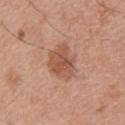Findings:
– notes — catalogued during a skin exam; not biopsied
– anatomic site — the upper back
– imaging modality — total-body-photography crop, ~15 mm field of view
– TBP lesion metrics — a border-irregularity rating of about 2.5/10 and radial color variation of about 1; a nevus-likeness score of about 50/100 and lesion-presence confidence of about 100/100
– patient — male, approximately 65 years of age
– lighting — white-light illumination
– lesion diameter — ~4.5 mm (longest diameter)